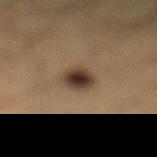Q: Is there a histopathology result?
A: catalogued during a skin exam; not biopsied
Q: What are the patient's age and sex?
A: male, in their mid- to late 30s
Q: What is the anatomic site?
A: the leg
Q: What is the lesion's diameter?
A: about 3.5 mm
Q: How was the tile lit?
A: cross-polarized
Q: What is the imaging modality?
A: ~15 mm crop, total-body skin-cancer survey